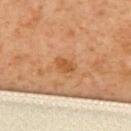Imaged during a routine full-body skin examination; the lesion was not biopsied and no histopathology is available.
A roughly 15 mm field-of-view crop from a total-body skin photograph.
Measured at roughly 2.5 mm in maximum diameter.
A female subject, in their mid- to late 50s.
An algorithmic analysis of the crop reported a lesion color around L≈57 a*≈25 b*≈42 in CIELAB, a lesion–skin lightness drop of about 9, and a normalized border contrast of about 6.5.
Captured under cross-polarized illumination.
The lesion is located on the upper back.